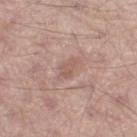Part of a total-body skin-imaging series; this lesion was reviewed on a skin check and was not flagged for biopsy. This is a white-light tile. A male patient aged 53 to 57. Measured at roughly 3.5 mm in maximum diameter. A 15 mm crop from a total-body photograph taken for skin-cancer surveillance. The lesion is located on the left thigh.